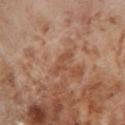notes: imaged on a skin check; not biopsied
diameter: ~3.5 mm (longest diameter)
body site: the right lower leg
lighting: cross-polarized
image: ~15 mm tile from a whole-body skin photo
TBP lesion metrics: a mean CIELAB color near L≈50 a*≈22 b*≈32 and a normalized lesion–skin contrast near 5.5
subject: female, aged approximately 55